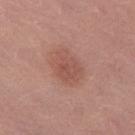Impression:
Part of a total-body skin-imaging series; this lesion was reviewed on a skin check and was not flagged for biopsy.
Image and clinical context:
Located on the right thigh. A female subject, roughly 65 years of age. The lesion's longest dimension is about 5 mm. This image is a 15 mm lesion crop taken from a total-body photograph. An algorithmic analysis of the crop reported a lesion area of about 13 mm², an outline eccentricity of about 0.75 (0 = round, 1 = elongated), and two-axis asymmetry of about 0.15. It also reported radial color variation of about 1. The software also gave a classifier nevus-likeness of about 90/100 and a lesion-detection confidence of about 100/100. This is a white-light tile.